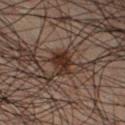Assessment: Recorded during total-body skin imaging; not selected for excision or biopsy. Background: The tile uses cross-polarized illumination. A male subject, about 50 years old. Longest diameter approximately 2.5 mm. A 15 mm crop from a total-body photograph taken for skin-cancer surveillance. The lesion is located on the right lower leg. Automated image analysis of the tile measured a footprint of about 5 mm², a shape eccentricity near 0.4, and two-axis asymmetry of about 0.3. It also reported internal color variation of about 2.5 on a 0–10 scale and a peripheral color-asymmetry measure near 1.